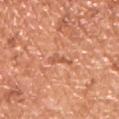Assessment:
The lesion was tiled from a total-body skin photograph and was not biopsied.
Image and clinical context:
The total-body-photography lesion software estimated a lesion color around L≈57 a*≈29 b*≈36 in CIELAB, about 9 CIELAB-L* units darker than the surrounding skin, and a normalized lesion–skin contrast near 5.5. A male subject aged around 55. Measured at roughly 3 mm in maximum diameter. A roughly 15 mm field-of-view crop from a total-body skin photograph. The lesion is on the chest.